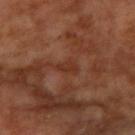follow-up: catalogued during a skin exam; not biopsied | patient: male, in their mid- to late 50s | site: the right upper arm | image: total-body-photography crop, ~15 mm field of view.